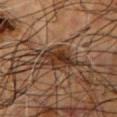Impression: The lesion was tiled from a total-body skin photograph and was not biopsied. Background: Captured under cross-polarized illumination. A male patient about 55 years old. A roughly 15 mm field-of-view crop from a total-body skin photograph. On the front of the torso. The lesion-visualizer software estimated a footprint of about 9.5 mm² and an outline eccentricity of about 0.75 (0 = round, 1 = elongated). The analysis additionally found roughly 10 lightness units darker than nearby skin and a normalized border contrast of about 10. It also reported internal color variation of about 6 on a 0–10 scale and peripheral color asymmetry of about 2. It also reported a classifier nevus-likeness of about 70/100. Approximately 4.5 mm at its widest.